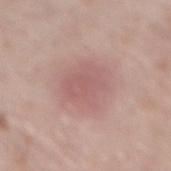Q: Is there a histopathology result?
A: catalogued during a skin exam; not biopsied